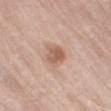Captured during whole-body skin photography for melanoma surveillance; the lesion was not biopsied. Longest diameter approximately 2.5 mm. From the left thigh. A male patient aged approximately 80. A lesion tile, about 15 mm wide, cut from a 3D total-body photograph. The lesion-visualizer software estimated a lesion area of about 6 mm², an eccentricity of roughly 0.4, and two-axis asymmetry of about 0.25. The software also gave a nevus-likeness score of about 65/100 and a detector confidence of about 100 out of 100 that the crop contains a lesion.